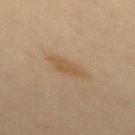The lesion was photographed on a routine skin check and not biopsied; there is no pathology result.
The patient is a female roughly 45 years of age.
Cropped from a whole-body photographic skin survey; the tile spans about 15 mm.
An algorithmic analysis of the crop reported an outline eccentricity of about 0.9 (0 = round, 1 = elongated).
From the upper back.
Captured under cross-polarized illumination.